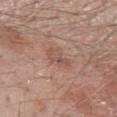<lesion>
<biopsy_status>not biopsied; imaged during a skin examination</biopsy_status>
<site>left forearm</site>
<automated_metrics>
  <cielab_L>53</cielab_L>
  <cielab_a>20</cielab_a>
  <cielab_b>26</cielab_b>
  <vs_skin_darker_L>6.0</vs_skin_darker_L>
</automated_metrics>
<lesion_size>
  <long_diameter_mm_approx>3.0</long_diameter_mm_approx>
</lesion_size>
<image>
  <source>total-body photography crop</source>
  <field_of_view_mm>15</field_of_view_mm>
</image>
<lighting>white-light</lighting>
<patient>
  <sex>male</sex>
  <age_approx>60</age_approx>
</patient>
</lesion>